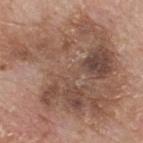No biopsy was performed on this lesion — it was imaged during a full skin examination and was not determined to be concerning. A 15 mm close-up tile from a total-body photography series done for melanoma screening. The lesion is located on the upper back. A male patient, approximately 80 years of age. This is a white-light tile.